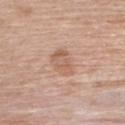biopsy_status: not biopsied; imaged during a skin examination
automated_metrics:
  eccentricity: 0.75
  shape_asymmetry: 0.2
  cielab_L: 60
  cielab_a: 20
  cielab_b: 30
  vs_skin_darker_L: 8.0
  border_irregularity_0_10: 2.0
  color_variation_0_10: 3.5
  peripheral_color_asymmetry: 1.0
  nevus_likeness_0_100: 0
  lesion_detection_confidence_0_100: 100
patient:
  sex: female
  age_approx: 65
image:
  source: total-body photography crop
  field_of_view_mm: 15
lighting: white-light
site: upper back
lesion_size:
  long_diameter_mm_approx: 4.0